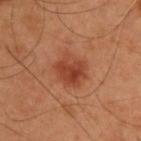A region of skin cropped from a whole-body photographic capture, roughly 15 mm wide. Measured at roughly 4 mm in maximum diameter. Imaged with cross-polarized lighting. From the upper back. The subject is a male aged approximately 55.